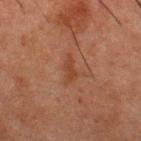Assessment:
The lesion was photographed on a routine skin check and not biopsied; there is no pathology result.
Acquisition and patient details:
A region of skin cropped from a whole-body photographic capture, roughly 15 mm wide. On the upper back. The total-body-photography lesion software estimated an area of roughly 3 mm², a shape eccentricity near 0.9, and two-axis asymmetry of about 0.35. And it measured an average lesion color of about L≈33 a*≈20 b*≈27 (CIELAB), roughly 6 lightness units darker than nearby skin, and a lesion-to-skin contrast of about 6 (normalized; higher = more distinct). And it measured a border-irregularity rating of about 4/10 and a color-variation rating of about 0/10. The analysis additionally found a classifier nevus-likeness of about 10/100 and lesion-presence confidence of about 100/100. A male patient roughly 50 years of age. The tile uses cross-polarized illumination.